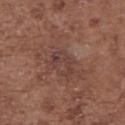Clinical summary: A female patient in their mid- to late 70s. A region of skin cropped from a whole-body photographic capture, roughly 15 mm wide. The recorded lesion diameter is about 3.5 mm. Imaged with white-light lighting. Located on the upper back.